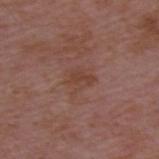Clinical impression: No biopsy was performed on this lesion — it was imaged during a full skin examination and was not determined to be concerning. Clinical summary: The subject is a male aged approximately 50. On the upper back. This image is a 15 mm lesion crop taken from a total-body photograph. Captured under white-light illumination.